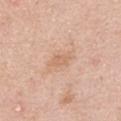  biopsy_status: not biopsied; imaged during a skin examination
  image:
    source: total-body photography crop
    field_of_view_mm: 15
  patient:
    sex: male
    age_approx: 50
  site: chest
  lesion_size:
    long_diameter_mm_approx: 2.5
  automated_metrics:
    cielab_L: 65
    cielab_a: 20
    cielab_b: 33
    vs_skin_contrast_norm: 5.0
    border_irregularity_0_10: 3.0
    peripheral_color_asymmetry: 0.5
    nevus_likeness_0_100: 0
    lesion_detection_confidence_0_100: 100